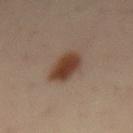{"biopsy_status": "not biopsied; imaged during a skin examination", "lighting": "cross-polarized", "lesion_size": {"long_diameter_mm_approx": 4.5}, "image": {"source": "total-body photography crop", "field_of_view_mm": 15}, "site": "mid back", "patient": {"sex": "male", "age_approx": 40}}